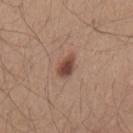A 15 mm crop from a total-body photograph taken for skin-cancer surveillance. A male patient, roughly 25 years of age. Approximately 3 mm at its widest. The lesion is on the mid back.